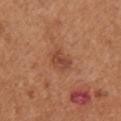<lesion>
<lighting>white-light</lighting>
<image>
  <source>total-body photography crop</source>
  <field_of_view_mm>15</field_of_view_mm>
</image>
<automated_metrics>
  <nevus_likeness_0_100>45</nevus_likeness_0_100>
  <lesion_detection_confidence_0_100>100</lesion_detection_confidence_0_100>
</automated_metrics>
<patient>
  <sex>female</sex>
  <age_approx>65</age_approx>
</patient>
<site>arm</site>
<lesion_size>
  <long_diameter_mm_approx>3.0</long_diameter_mm_approx>
</lesion_size>
</lesion>